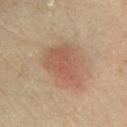Q: Was this lesion biopsied?
A: no biopsy performed (imaged during a skin exam)
Q: How was this image acquired?
A: total-body-photography crop, ~15 mm field of view
Q: Where on the body is the lesion?
A: the right lower leg
Q: What are the patient's age and sex?
A: female, roughly 55 years of age
Q: How large is the lesion?
A: ≈5 mm
Q: How was the tile lit?
A: cross-polarized illumination